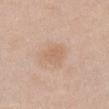Clinical impression: This lesion was catalogued during total-body skin photography and was not selected for biopsy. Image and clinical context: The patient is a female aged 48–52. The recorded lesion diameter is about 2.5 mm. From the chest. The lesion-visualizer software estimated a border-irregularity rating of about 3/10, a within-lesion color-variation index near 1/10, and radial color variation of about 0.5. The software also gave an automated nevus-likeness rating near 0 out of 100 and lesion-presence confidence of about 100/100. A 15 mm close-up tile from a total-body photography series done for melanoma screening.